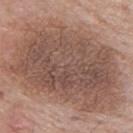Captured under white-light illumination.
A male subject, aged 68–72.
Approximately 14 mm at its widest.
This image is a 15 mm lesion crop taken from a total-body photograph.
Located on the upper back.
The total-body-photography lesion software estimated a lesion area of about 95 mm², a shape eccentricity near 0.75, and a symmetry-axis asymmetry near 0.2. It also reported border irregularity of about 3.5 on a 0–10 scale, a within-lesion color-variation index near 5.5/10, and radial color variation of about 2. The analysis additionally found an automated nevus-likeness rating near 0 out of 100 and a detector confidence of about 100 out of 100 that the crop contains a lesion.